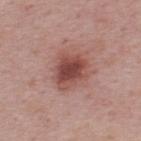Imaged during a routine full-body skin examination; the lesion was not biopsied and no histopathology is available. A male subject roughly 50 years of age. The lesion is located on the back. Cropped from a whole-body photographic skin survey; the tile spans about 15 mm.